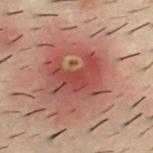The lesion was photographed on a routine skin check and not biopsied; there is no pathology result. From the chest. The recorded lesion diameter is about 5.5 mm. A male subject in their 30s. An algorithmic analysis of the crop reported an area of roughly 16 mm² and an eccentricity of roughly 0.75. It also reported a lesion color around L≈37 a*≈25 b*≈22 in CIELAB, about 8 CIELAB-L* units darker than the surrounding skin, and a normalized lesion–skin contrast near 6.5. It also reported a classifier nevus-likeness of about 0/100 and a detector confidence of about 100 out of 100 that the crop contains a lesion. A 15 mm close-up tile from a total-body photography series done for melanoma screening.